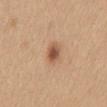Part of a total-body skin-imaging series; this lesion was reviewed on a skin check and was not flagged for biopsy. A region of skin cropped from a whole-body photographic capture, roughly 15 mm wide. This is a white-light tile. Located on the back. A female subject aged around 45.